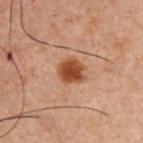{
  "patient": {
    "sex": "male",
    "age_approx": 60
  },
  "lesion_size": {
    "long_diameter_mm_approx": 3.0
  },
  "image": {
    "source": "total-body photography crop",
    "field_of_view_mm": 15
  },
  "site": "chest",
  "automated_metrics": {
    "cielab_L": 35,
    "cielab_a": 21,
    "cielab_b": 28,
    "vs_skin_darker_L": 12.0,
    "vs_skin_contrast_norm": 11.0,
    "nevus_likeness_0_100": 100,
    "lesion_detection_confidence_0_100": 100
  },
  "lighting": "cross-polarized"
}